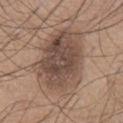Impression: No biopsy was performed on this lesion — it was imaged during a full skin examination and was not determined to be concerning. Image and clinical context: An algorithmic analysis of the crop reported an area of roughly 39 mm², a shape eccentricity near 0.75, and a shape-asymmetry score of about 0.15 (0 = symmetric). The software also gave an average lesion color of about L≈48 a*≈16 b*≈25 (CIELAB). It also reported an automated nevus-likeness rating near 5 out of 100 and lesion-presence confidence of about 100/100. The patient is a male approximately 75 years of age. Measured at roughly 8.5 mm in maximum diameter. A lesion tile, about 15 mm wide, cut from a 3D total-body photograph. Located on the abdomen.